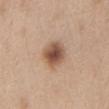{
  "biopsy_status": "not biopsied; imaged during a skin examination",
  "lesion_size": {
    "long_diameter_mm_approx": 3.5
  },
  "image": {
    "source": "total-body photography crop",
    "field_of_view_mm": 15
  },
  "automated_metrics": {
    "cielab_L": 53,
    "cielab_a": 19,
    "cielab_b": 30,
    "vs_skin_darker_L": 14.0,
    "vs_skin_contrast_norm": 9.5,
    "border_irregularity_0_10": 1.0,
    "color_variation_0_10": 5.5,
    "nevus_likeness_0_100": 100
  },
  "patient": {
    "sex": "female",
    "age_approx": 40
  },
  "site": "abdomen",
  "lighting": "white-light"
}